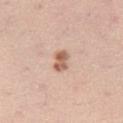Assessment:
Part of a total-body skin-imaging series; this lesion was reviewed on a skin check and was not flagged for biopsy.
Context:
A roughly 15 mm field-of-view crop from a total-body skin photograph. The recorded lesion diameter is about 2.5 mm. The subject is a female aged around 40. Imaged with white-light lighting. From the left thigh.